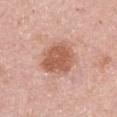Impression:
The lesion was tiled from a total-body skin photograph and was not biopsied.
Context:
The patient is a female aged around 50. From the arm. The total-body-photography lesion software estimated about 12 CIELAB-L* units darker than the surrounding skin and a lesion-to-skin contrast of about 8 (normalized; higher = more distinct). It also reported a nevus-likeness score of about 65/100 and a lesion-detection confidence of about 100/100. The lesion's longest dimension is about 4.5 mm. This is a white-light tile. A 15 mm close-up extracted from a 3D total-body photography capture.